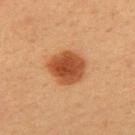The lesion was tiled from a total-body skin photograph and was not biopsied. A female subject aged 38 to 42. This is a cross-polarized tile. Located on the left upper arm. The recorded lesion diameter is about 4 mm. Cropped from a total-body skin-imaging series; the visible field is about 15 mm.